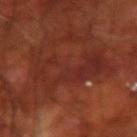{"biopsy_status": "not biopsied; imaged during a skin examination", "patient": {"sex": "male", "age_approx": 70}, "site": "right forearm", "lesion_size": {"long_diameter_mm_approx": 9.0}, "image": {"source": "total-body photography crop", "field_of_view_mm": 15}, "lighting": "cross-polarized"}